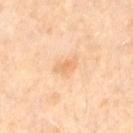The lesion was tiled from a total-body skin photograph and was not biopsied. The lesion is on the right thigh. A lesion tile, about 15 mm wide, cut from a 3D total-body photograph. The patient is a female aged approximately 50. Imaged with cross-polarized lighting.